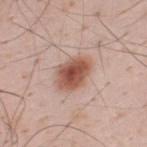follow-up: no biopsy performed (imaged during a skin exam).